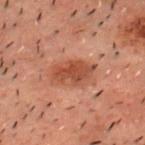Assessment:
No biopsy was performed on this lesion — it was imaged during a full skin examination and was not determined to be concerning.